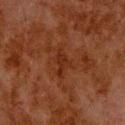Imaged during a routine full-body skin examination; the lesion was not biopsied and no histopathology is available.
A male patient, aged approximately 80.
Automated tile analysis of the lesion measured a footprint of about 5 mm², a shape eccentricity near 0.85, and a shape-asymmetry score of about 0.4 (0 = symmetric). The analysis additionally found a color-variation rating of about 2/10 and radial color variation of about 0.5. The software also gave a nevus-likeness score of about 0/100 and a detector confidence of about 100 out of 100 that the crop contains a lesion.
On the upper back.
About 4 mm across.
Cropped from a total-body skin-imaging series; the visible field is about 15 mm.
Captured under cross-polarized illumination.